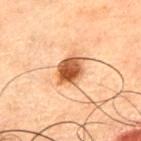{
  "biopsy_status": "not biopsied; imaged during a skin examination",
  "lesion_size": {
    "long_diameter_mm_approx": 4.0
  },
  "image": {
    "source": "total-body photography crop",
    "field_of_view_mm": 15
  },
  "site": "chest",
  "automated_metrics": {
    "cielab_L": 44,
    "cielab_a": 21,
    "cielab_b": 32,
    "vs_skin_darker_L": 15.0,
    "vs_skin_contrast_norm": 11.5
  },
  "patient": {
    "sex": "male",
    "age_approx": 50
  }
}